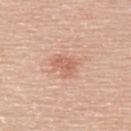| key | value |
|---|---|
| follow-up | no biopsy performed (imaged during a skin exam) |
| tile lighting | white-light illumination |
| size | ≈3 mm |
| TBP lesion metrics | a footprint of about 5 mm² and an outline eccentricity of about 0.65 (0 = round, 1 = elongated); a lesion color around L≈62 a*≈24 b*≈31 in CIELAB, a lesion–skin lightness drop of about 9, and a normalized lesion–skin contrast near 6; a border-irregularity index near 3/10, a color-variation rating of about 3/10, and peripheral color asymmetry of about 1; a classifier nevus-likeness of about 10/100 and lesion-presence confidence of about 100/100 |
| image | total-body-photography crop, ~15 mm field of view |
| subject | male, approximately 60 years of age |
| anatomic site | the upper back |